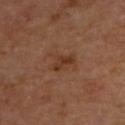A 15 mm close-up tile from a total-body photography series done for melanoma screening.
Captured under cross-polarized illumination.
A patient aged around 65.
From the upper back.
About 3 mm across.